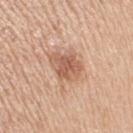notes=total-body-photography surveillance lesion; no biopsy | anatomic site=the right upper arm | subject=female, aged approximately 55 | image source=total-body-photography crop, ~15 mm field of view.